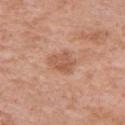Impression: Imaged during a routine full-body skin examination; the lesion was not biopsied and no histopathology is available. Clinical summary: The tile uses white-light illumination. A female patient roughly 70 years of age. About 3 mm across. A region of skin cropped from a whole-body photographic capture, roughly 15 mm wide. From the right upper arm. The lesion-visualizer software estimated a lesion–skin lightness drop of about 9 and a normalized lesion–skin contrast near 6.